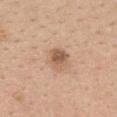size: ~2.5 mm (longest diameter); subject: female, in their mid-40s; illumination: white-light; imaging modality: 15 mm crop, total-body photography; location: the upper back.